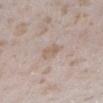No biopsy was performed on this lesion — it was imaged during a full skin examination and was not determined to be concerning. Measured at roughly 3 mm in maximum diameter. The tile uses white-light illumination. A female subject, aged 23–27. A lesion tile, about 15 mm wide, cut from a 3D total-body photograph. On the left lower leg.